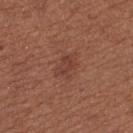Case summary:
- follow-up: total-body-photography surveillance lesion; no biopsy
- lighting: white-light illumination
- lesion size: ≈3 mm
- TBP lesion metrics: an area of roughly 5 mm², an eccentricity of roughly 0.75, and a shape-asymmetry score of about 0.3 (0 = symmetric); internal color variation of about 1.5 on a 0–10 scale and a peripheral color-asymmetry measure near 0.5; a classifier nevus-likeness of about 15/100 and a detector confidence of about 100 out of 100 that the crop contains a lesion
- image source: ~15 mm crop, total-body skin-cancer survey
- patient: female, roughly 55 years of age
- body site: the leg